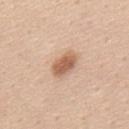Impression:
This lesion was catalogued during total-body skin photography and was not selected for biopsy.
Acquisition and patient details:
Located on the mid back. The subject is a male about 45 years old. This is a white-light tile. A 15 mm close-up tile from a total-body photography series done for melanoma screening. Automated image analysis of the tile measured a shape eccentricity near 0.8 and a shape-asymmetry score of about 0.25 (0 = symmetric). The software also gave a border-irregularity rating of about 2.5/10, a color-variation rating of about 3/10, and radial color variation of about 1. The software also gave an automated nevus-likeness rating near 95 out of 100.